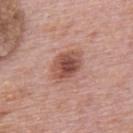Q: Was this lesion biopsied?
A: total-body-photography surveillance lesion; no biopsy
Q: What is the lesion's diameter?
A: ~4 mm (longest diameter)
Q: How was this image acquired?
A: ~15 mm tile from a whole-body skin photo
Q: What did automated image analysis measure?
A: a lesion area of about 10 mm² and a shape eccentricity near 0.65; a border-irregularity rating of about 1.5/10, internal color variation of about 5.5 on a 0–10 scale, and a peripheral color-asymmetry measure near 1.5
Q: Patient demographics?
A: male, aged around 75
Q: Lesion location?
A: the upper back
Q: Illumination type?
A: white-light illumination